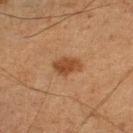The lesion was photographed on a routine skin check and not biopsied; there is no pathology result. On the left lower leg. A close-up tile cropped from a whole-body skin photograph, about 15 mm across. A male patient aged approximately 75.